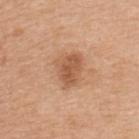The subject is a female aged 28–32. Located on the upper back. The recorded lesion diameter is about 3.5 mm. The lesion-visualizer software estimated a lesion area of about 9 mm² and a shape eccentricity near 0.6. The analysis additionally found a border-irregularity rating of about 3/10 and radial color variation of about 1. It also reported an automated nevus-likeness rating near 65 out of 100. A 15 mm crop from a total-body photograph taken for skin-cancer surveillance.